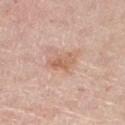Imaged during a routine full-body skin examination; the lesion was not biopsied and no histopathology is available. The lesion is located on the right thigh. Longest diameter approximately 3 mm. The lesion-visualizer software estimated an average lesion color of about L≈62 a*≈21 b*≈31 (CIELAB) and a normalized border contrast of about 6.5. The analysis additionally found a classifier nevus-likeness of about 10/100. A close-up tile cropped from a whole-body skin photograph, about 15 mm across. A female subject in their mid- to late 60s. The tile uses white-light illumination.